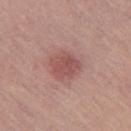This lesion was catalogued during total-body skin photography and was not selected for biopsy. Captured under white-light illumination. A female patient approximately 50 years of age. The recorded lesion diameter is about 3.5 mm. A 15 mm crop from a total-body photograph taken for skin-cancer surveillance. The lesion is located on the left thigh.